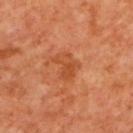Impression: Part of a total-body skin-imaging series; this lesion was reviewed on a skin check and was not flagged for biopsy. Background: A 15 mm close-up extracted from a 3D total-body photography capture. Automated image analysis of the tile measured a border-irregularity rating of about 5/10, a color-variation rating of about 4/10, and a peripheral color-asymmetry measure near 1.5. A female subject aged 38 to 42. On the upper back. Captured under cross-polarized illumination. The lesion's longest dimension is about 3.5 mm.